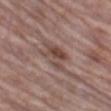The lesion was tiled from a total-body skin photograph and was not biopsied. Imaged with white-light lighting. A male subject aged around 70. The lesion's longest dimension is about 5 mm. Automated image analysis of the tile measured a normalized border contrast of about 7. A lesion tile, about 15 mm wide, cut from a 3D total-body photograph. Located on the left thigh.